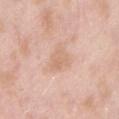Assessment: The lesion was photographed on a routine skin check and not biopsied; there is no pathology result. Acquisition and patient details: A male subject roughly 55 years of age. An algorithmic analysis of the crop reported an area of roughly 6 mm². It also reported a mean CIELAB color near L≈68 a*≈19 b*≈31, roughly 7 lightness units darker than nearby skin, and a lesion-to-skin contrast of about 5 (normalized; higher = more distinct). And it measured a classifier nevus-likeness of about 0/100 and lesion-presence confidence of about 100/100. Cropped from a whole-body photographic skin survey; the tile spans about 15 mm. From the abdomen.